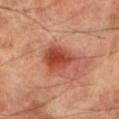Clinical impression: The lesion was photographed on a routine skin check and not biopsied; there is no pathology result. Image and clinical context: Longest diameter approximately 5 mm. The lesion-visualizer software estimated an area of roughly 12 mm² and an outline eccentricity of about 0.65 (0 = round, 1 = elongated). And it measured a mean CIELAB color near L≈48 a*≈30 b*≈33, a lesion–skin lightness drop of about 12, and a lesion-to-skin contrast of about 8.5 (normalized; higher = more distinct). A male patient aged 68 to 72. A close-up tile cropped from a whole-body skin photograph, about 15 mm across. Located on the left lower leg. This is a cross-polarized tile.